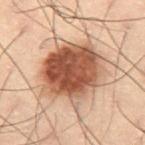The lesion was photographed on a routine skin check and not biopsied; there is no pathology result.
Captured under cross-polarized illumination.
The lesion's longest dimension is about 6.5 mm.
A male subject, aged 53 to 57.
Automated tile analysis of the lesion measured a footprint of about 33 mm², an outline eccentricity of about 0.35 (0 = round, 1 = elongated), and a shape-asymmetry score of about 0.15 (0 = symmetric). The software also gave a classifier nevus-likeness of about 95/100 and a lesion-detection confidence of about 100/100.
On the right thigh.
A roughly 15 mm field-of-view crop from a total-body skin photograph.